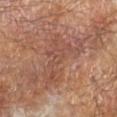  lesion_size:
    long_diameter_mm_approx: 6.5
  lighting: cross-polarized
  site: left forearm
  image:
    source: total-body photography crop
    field_of_view_mm: 15
  patient:
    sex: male
    age_approx: 65